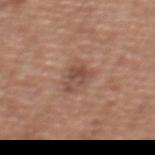Clinical impression:
The lesion was photographed on a routine skin check and not biopsied; there is no pathology result.
Context:
A 15 mm crop from a total-body photograph taken for skin-cancer surveillance. The patient is a female aged approximately 60. Approximately 3 mm at its widest. Imaged with white-light lighting. The lesion is located on the upper back.